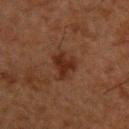Impression: This lesion was catalogued during total-body skin photography and was not selected for biopsy. Clinical summary: Imaged with cross-polarized lighting. About 3 mm across. A 15 mm close-up tile from a total-body photography series done for melanoma screening. On the upper back. The subject is a male in their 50s.